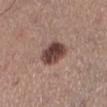Q: Was a biopsy performed?
A: no biopsy performed (imaged during a skin exam)